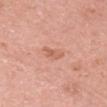No biopsy was performed on this lesion — it was imaged during a full skin examination and was not determined to be concerning.
From the head or neck.
A female subject aged approximately 60.
A roughly 15 mm field-of-view crop from a total-body skin photograph.
Automated tile analysis of the lesion measured a mean CIELAB color near L≈61 a*≈26 b*≈32, roughly 8 lightness units darker than nearby skin, and a lesion-to-skin contrast of about 5.5 (normalized; higher = more distinct). It also reported border irregularity of about 3.5 on a 0–10 scale, a color-variation rating of about 2.5/10, and a peripheral color-asymmetry measure near 1.
The tile uses white-light illumination.
Measured at roughly 2.5 mm in maximum diameter.